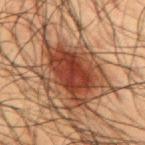patient=male, roughly 60 years of age
lesion size=~8 mm (longest diameter)
location=the mid back
lighting=cross-polarized illumination
image source=total-body-photography crop, ~15 mm field of view
automated lesion analysis=a lesion color around L≈32 a*≈22 b*≈26 in CIELAB and roughly 13 lightness units darker than nearby skin; a border-irregularity index near 4.5/10, a color-variation rating of about 4.5/10, and a peripheral color-asymmetry measure near 1.5